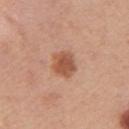  lesion_size:
    long_diameter_mm_approx: 3.5
  lighting: white-light
  patient:
    sex: female
    age_approx: 45
  automated_metrics:
    border_irregularity_0_10: 1.5
    color_variation_0_10: 3.5
    peripheral_color_asymmetry: 1.0
    nevus_likeness_0_100: 95
    lesion_detection_confidence_0_100: 100
  image:
    source: total-body photography crop
    field_of_view_mm: 15
  site: left upper arm